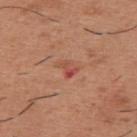Recorded during total-body skin imaging; not selected for excision or biopsy.
This is a white-light tile.
A male subject, aged 38 to 42.
The lesion's longest dimension is about 2.5 mm.
The lesion is located on the upper back.
Cropped from a total-body skin-imaging series; the visible field is about 15 mm.
Automated image analysis of the tile measured an area of roughly 3.5 mm², an outline eccentricity of about 0.75 (0 = round, 1 = elongated), and two-axis asymmetry of about 0.4. It also reported a border-irregularity rating of about 3.5/10, internal color variation of about 8.5 on a 0–10 scale, and peripheral color asymmetry of about 3. And it measured an automated nevus-likeness rating near 0 out of 100 and lesion-presence confidence of about 100/100.